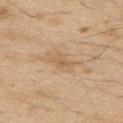Recorded during total-body skin imaging; not selected for excision or biopsy.
About 2.5 mm across.
A male patient aged 68 to 72.
The tile uses white-light illumination.
This image is a 15 mm lesion crop taken from a total-body photograph.
From the upper back.